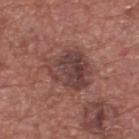This lesion was catalogued during total-body skin photography and was not selected for biopsy.